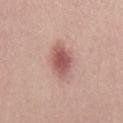tile lighting = white-light | subject = male, aged 28 to 32 | imaging modality = ~15 mm tile from a whole-body skin photo | diameter = ≈5 mm.